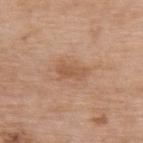This lesion was catalogued during total-body skin photography and was not selected for biopsy. This is a white-light tile. From the upper back. A close-up tile cropped from a whole-body skin photograph, about 15 mm across. The patient is a female aged 73–77.